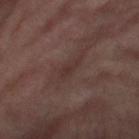The lesion was tiled from a total-body skin photograph and was not biopsied. The lesion is on the right thigh. This is a cross-polarized tile. Longest diameter approximately 2.5 mm. The lesion-visualizer software estimated a mean CIELAB color near L≈32 a*≈17 b*≈19, a lesion–skin lightness drop of about 5, and a lesion-to-skin contrast of about 5.5 (normalized; higher = more distinct). The analysis additionally found border irregularity of about 5 on a 0–10 scale. A roughly 15 mm field-of-view crop from a total-body skin photograph. A female subject approximately 55 years of age.